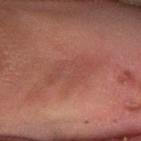biopsy status: imaged on a skin check; not biopsied | diameter: ≈6 mm | TBP lesion metrics: border irregularity of about 4 on a 0–10 scale, internal color variation of about 2.5 on a 0–10 scale, and peripheral color asymmetry of about 1 | subject: female, in their 80s | imaging modality: ~15 mm tile from a whole-body skin photo | location: the right lower leg.